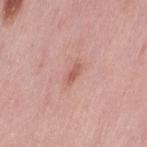notes: imaged on a skin check; not biopsied | anatomic site: the leg | imaging modality: total-body-photography crop, ~15 mm field of view | subject: female, approximately 50 years of age.